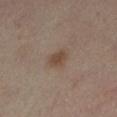follow-up: total-body-photography surveillance lesion; no biopsy | size: ~2.5 mm (longest diameter) | lighting: cross-polarized | site: the right leg | image: ~15 mm tile from a whole-body skin photo | subject: female, aged 38 to 42 | automated lesion analysis: an outline eccentricity of about 0.7 (0 = round, 1 = elongated) and a symmetry-axis asymmetry near 0.25; an average lesion color of about L≈45 a*≈15 b*≈27 (CIELAB), a lesion–skin lightness drop of about 8, and a normalized border contrast of about 7.5; a within-lesion color-variation index near 2/10 and radial color variation of about 0.5.